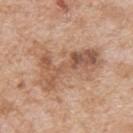follow-up: imaged on a skin check; not biopsied
illumination: white-light illumination
imaging modality: ~15 mm crop, total-body skin-cancer survey
subject: male, in their mid-60s
body site: the upper back
diameter: ≈7.5 mm
TBP lesion metrics: a lesion area of about 17 mm² and two-axis asymmetry of about 0.5; a mean CIELAB color near L≈55 a*≈20 b*≈31, roughly 11 lightness units darker than nearby skin, and a normalized lesion–skin contrast near 7.5; a lesion-detection confidence of about 100/100